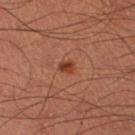{"biopsy_status": "not biopsied; imaged during a skin examination", "lesion_size": {"long_diameter_mm_approx": 2.0}, "lighting": "cross-polarized", "site": "right thigh", "automated_metrics": {"border_irregularity_0_10": 1.5, "color_variation_0_10": 3.0, "peripheral_color_asymmetry": 1.0}, "patient": {"sex": "male", "age_approx": 35}, "image": {"source": "total-body photography crop", "field_of_view_mm": 15}}